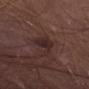Impression: Imaged during a routine full-body skin examination; the lesion was not biopsied and no histopathology is available. Background: A male subject in their 40s. On the abdomen. A roughly 15 mm field-of-view crop from a total-body skin photograph.